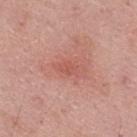notes — catalogued during a skin exam; not biopsied
anatomic site — the back
patient — male, aged approximately 45
lesion diameter — ~2 mm (longest diameter)
tile lighting — white-light illumination
image source — ~15 mm tile from a whole-body skin photo